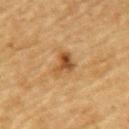Assessment: The lesion was photographed on a routine skin check and not biopsied; there is no pathology result. Acquisition and patient details: From the upper back. Approximately 3 mm at its widest. A male patient, in their mid- to late 80s or older. A close-up tile cropped from a whole-body skin photograph, about 15 mm across. Automated tile analysis of the lesion measured an area of roughly 5.5 mm², an outline eccentricity of about 0.7 (0 = round, 1 = elongated), and a shape-asymmetry score of about 0.45 (0 = symmetric). It also reported a within-lesion color-variation index near 5/10 and a peripheral color-asymmetry measure near 1.5. Imaged with cross-polarized lighting.